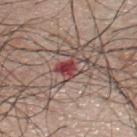imaging modality — ~15 mm crop, total-body skin-cancer survey | automated lesion analysis — a lesion color around L≈44 a*≈27 b*≈22 in CIELAB, roughly 12 lightness units darker than nearby skin, and a lesion-to-skin contrast of about 9.5 (normalized; higher = more distinct); a border-irregularity rating of about 5.5/10, a within-lesion color-variation index near 4.5/10, and a peripheral color-asymmetry measure near 1.5; a classifier nevus-likeness of about 0/100 and lesion-presence confidence of about 100/100 | illumination — white-light | site — the back | patient — male, aged 43–47.